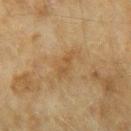This lesion was catalogued during total-body skin photography and was not selected for biopsy.
This image is a 15 mm lesion crop taken from a total-body photograph.
The subject is a female aged around 60.
Captured under cross-polarized illumination.
Longest diameter approximately 3.5 mm.
Located on the arm.